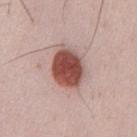Clinical impression: Recorded during total-body skin imaging; not selected for excision or biopsy. Context: Imaged with white-light lighting. The lesion is located on the chest. The lesion's longest dimension is about 5 mm. The lesion-visualizer software estimated a lesion area of about 14 mm², a shape eccentricity near 0.65, and two-axis asymmetry of about 0.1. It also reported a classifier nevus-likeness of about 100/100 and a detector confidence of about 100 out of 100 that the crop contains a lesion. This image is a 15 mm lesion crop taken from a total-body photograph. A male subject, aged 33 to 37.